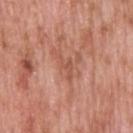| key | value |
|---|---|
| notes | total-body-photography surveillance lesion; no biopsy |
| body site | the head or neck |
| image | 15 mm crop, total-body photography |
| tile lighting | white-light illumination |
| size | ~3.5 mm (longest diameter) |
| subject | male, aged around 60 |